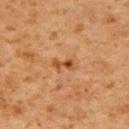Impression: Imaged during a routine full-body skin examination; the lesion was not biopsied and no histopathology is available. Image and clinical context: A region of skin cropped from a whole-body photographic capture, roughly 15 mm wide. On the right upper arm. Measured at roughly 2.5 mm in maximum diameter. The tile uses cross-polarized illumination. Automated tile analysis of the lesion measured a lesion area of about 2.5 mm², an outline eccentricity of about 0.9 (0 = round, 1 = elongated), and a symmetry-axis asymmetry near 0.45. The analysis additionally found an average lesion color of about L≈41 a*≈22 b*≈35 (CIELAB), roughly 10 lightness units darker than nearby skin, and a lesion-to-skin contrast of about 8.5 (normalized; higher = more distinct). The software also gave border irregularity of about 5 on a 0–10 scale and a peripheral color-asymmetry measure near 0. It also reported a nevus-likeness score of about 45/100. A male subject, about 60 years old.